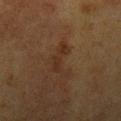notes — no biopsy performed (imaged during a skin exam) | image source — total-body-photography crop, ~15 mm field of view | patient — female, about 55 years old | location — the left upper arm.